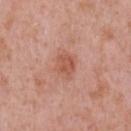This lesion was catalogued during total-body skin photography and was not selected for biopsy. The tile uses white-light illumination. The lesion's longest dimension is about 3 mm. Cropped from a whole-body photographic skin survey; the tile spans about 15 mm. A male patient, roughly 50 years of age. The lesion is on the chest. An algorithmic analysis of the crop reported a footprint of about 5.5 mm² and a shape-asymmetry score of about 0.25 (0 = symmetric). And it measured a lesion color around L≈55 a*≈26 b*≈30 in CIELAB, about 9 CIELAB-L* units darker than the surrounding skin, and a normalized lesion–skin contrast near 6.5. And it measured a border-irregularity rating of about 2.5/10, internal color variation of about 2 on a 0–10 scale, and peripheral color asymmetry of about 0.5. And it measured a classifier nevus-likeness of about 20/100 and lesion-presence confidence of about 100/100.